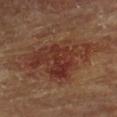biopsy status: catalogued during a skin exam; not biopsied | lesion size: about 9.5 mm | subject: female, aged around 80 | TBP lesion metrics: a mean CIELAB color near L≈30 a*≈21 b*≈24, roughly 7 lightness units darker than nearby skin, and a lesion-to-skin contrast of about 7.5 (normalized; higher = more distinct); a border-irregularity index near 7/10 and radial color variation of about 1.5; a nevus-likeness score of about 15/100 and lesion-presence confidence of about 100/100 | image: total-body-photography crop, ~15 mm field of view | location: the chest.